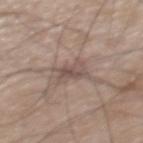Part of a total-body skin-imaging series; this lesion was reviewed on a skin check and was not flagged for biopsy. The lesion is on the back. The tile uses white-light illumination. The recorded lesion diameter is about 3.5 mm. A male patient roughly 75 years of age. Automated tile analysis of the lesion measured an automated nevus-likeness rating near 0 out of 100. Cropped from a whole-body photographic skin survey; the tile spans about 15 mm.